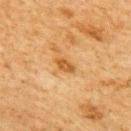<record>
  <biopsy_status>not biopsied; imaged during a skin examination</biopsy_status>
  <lesion_size>
    <long_diameter_mm_approx>2.5</long_diameter_mm_approx>
  </lesion_size>
  <lighting>cross-polarized</lighting>
  <patient>
    <sex>female</sex>
    <age_approx>60</age_approx>
  </patient>
  <image>
    <source>total-body photography crop</source>
    <field_of_view_mm>15</field_of_view_mm>
  </image>
  <site>upper back</site>
</record>